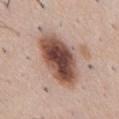Clinical impression:
This lesion was catalogued during total-body skin photography and was not selected for biopsy.
Image and clinical context:
About 7.5 mm across. The lesion is located on the abdomen. A male patient, about 50 years old. Automated image analysis of the tile measured about 19 CIELAB-L* units darker than the surrounding skin and a lesion-to-skin contrast of about 12.5 (normalized; higher = more distinct). And it measured a border-irregularity index near 2/10 and internal color variation of about 9.5 on a 0–10 scale. And it measured a nevus-likeness score of about 95/100 and a lesion-detection confidence of about 100/100. A region of skin cropped from a whole-body photographic capture, roughly 15 mm wide. Captured under white-light illumination.